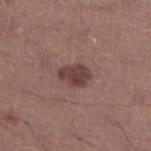workup = imaged on a skin check; not biopsied
TBP lesion metrics = an area of roughly 6.5 mm²; a lesion color around L≈41 a*≈19 b*≈20 in CIELAB, about 11 CIELAB-L* units darker than the surrounding skin, and a normalized lesion–skin contrast near 9; a nevus-likeness score of about 65/100 and lesion-presence confidence of about 100/100
tile lighting = white-light illumination
subject = male, about 45 years old
anatomic site = the left lower leg
imaging modality = total-body-photography crop, ~15 mm field of view